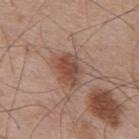notes = imaged on a skin check; not biopsied
site = the mid back
acquisition = 15 mm crop, total-body photography
patient = male, aged around 55
illumination = white-light illumination